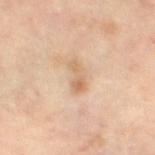<case>
<biopsy_status>not biopsied; imaged during a skin examination</biopsy_status>
<lighting>cross-polarized</lighting>
<automated_metrics>
  <cielab_L>64</cielab_L>
  <cielab_a>18</cielab_a>
  <cielab_b>33</cielab_b>
  <vs_skin_darker_L>9.0</vs_skin_darker_L>
  <vs_skin_contrast_norm>6.0</vs_skin_contrast_norm>
  <nevus_likeness_0_100>10</nevus_likeness_0_100>
  <lesion_detection_confidence_0_100>100</lesion_detection_confidence_0_100>
</automated_metrics>
<patient>
  <sex>female</sex>
  <age_approx>65</age_approx>
</patient>
<image>
  <source>total-body photography crop</source>
  <field_of_view_mm>15</field_of_view_mm>
</image>
<lesion_size>
  <long_diameter_mm_approx>3.5</long_diameter_mm_approx>
</lesion_size>
<site>leg</site>
</case>